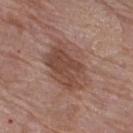Recorded during total-body skin imaging; not selected for excision or biopsy. A 15 mm crop from a total-body photograph taken for skin-cancer surveillance. The tile uses white-light illumination. The patient is a female aged 68–72. From the right thigh. Automated tile analysis of the lesion measured a lesion area of about 23 mm², a shape eccentricity near 0.7, and a shape-asymmetry score of about 0.2 (0 = symmetric). The analysis additionally found about 9 CIELAB-L* units darker than the surrounding skin and a lesion-to-skin contrast of about 7 (normalized; higher = more distinct). The software also gave a nevus-likeness score of about 40/100 and a lesion-detection confidence of about 100/100. The recorded lesion diameter is about 6.5 mm.